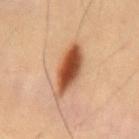Q: Where on the body is the lesion?
A: the mid back
Q: Patient demographics?
A: male, aged around 55
Q: How was this image acquired?
A: 15 mm crop, total-body photography
Q: What lighting was used for the tile?
A: cross-polarized
Q: Lesion size?
A: ~6 mm (longest diameter)